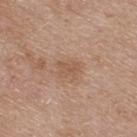biopsy_status: not biopsied; imaged during a skin examination
site: upper back
automated_metrics:
  eccentricity: 0.75
  shape_asymmetry: 0.35
lesion_size:
  long_diameter_mm_approx: 2.5
image:
  source: total-body photography crop
  field_of_view_mm: 15
lighting: white-light
patient:
  sex: male
  age_approx: 75